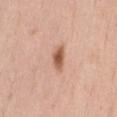biopsy status — catalogued during a skin exam; not biopsied
imaging modality — 15 mm crop, total-body photography
anatomic site — the abdomen
lesion diameter — ~3 mm (longest diameter)
patient — female, about 50 years old
illumination — white-light